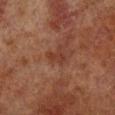Impression: Part of a total-body skin-imaging series; this lesion was reviewed on a skin check and was not flagged for biopsy. Image and clinical context: On the right lower leg. The lesion's longest dimension is about 2.5 mm. A close-up tile cropped from a whole-body skin photograph, about 15 mm across. A male subject roughly 70 years of age.